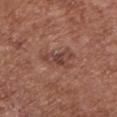Notes:
– image — total-body-photography crop, ~15 mm field of view
– location — the front of the torso
– tile lighting — white-light illumination
– size — ≈4 mm
– patient — female, aged 73–77
– TBP lesion metrics — a border-irregularity rating of about 6/10, a within-lesion color-variation index near 3/10, and a peripheral color-asymmetry measure near 0.5; an automated nevus-likeness rating near 0 out of 100 and a lesion-detection confidence of about 100/100